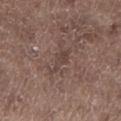Recorded during total-body skin imaging; not selected for excision or biopsy. The lesion is on the left lower leg. The lesion-visualizer software estimated a lesion area of about 4 mm² and a shape-asymmetry score of about 0.45 (0 = symmetric). The software also gave a mean CIELAB color near L≈42 a*≈16 b*≈21, a lesion–skin lightness drop of about 6, and a lesion-to-skin contrast of about 5.5 (normalized; higher = more distinct). A male subject aged around 70. Cropped from a whole-body photographic skin survey; the tile spans about 15 mm. The recorded lesion diameter is about 3.5 mm. The tile uses white-light illumination.